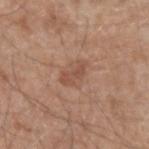The lesion was tiled from a total-body skin photograph and was not biopsied. The lesion-visualizer software estimated an area of roughly 5 mm². The analysis additionally found an average lesion color of about L≈51 a*≈21 b*≈29 (CIELAB) and about 8 CIELAB-L* units darker than the surrounding skin. The analysis additionally found a border-irregularity rating of about 4/10, internal color variation of about 1.5 on a 0–10 scale, and peripheral color asymmetry of about 0.5. On the left forearm. This is a white-light tile. A male subject, aged approximately 60. A 15 mm close-up tile from a total-body photography series done for melanoma screening.